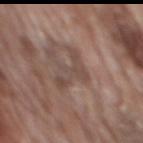This lesion was catalogued during total-body skin photography and was not selected for biopsy. A male patient aged approximately 70. Captured under white-light illumination. The lesion is located on the back. Longest diameter approximately 5 mm. A roughly 15 mm field-of-view crop from a total-body skin photograph.